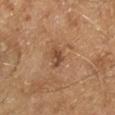Recorded during total-body skin imaging; not selected for excision or biopsy. A male subject, roughly 65 years of age. The recorded lesion diameter is about 2 mm. The total-body-photography lesion software estimated an eccentricity of roughly 0.65 and two-axis asymmetry of about 0.25. The analysis additionally found a mean CIELAB color near L≈46 a*≈20 b*≈32, a lesion–skin lightness drop of about 10, and a lesion-to-skin contrast of about 7.5 (normalized; higher = more distinct). It also reported a color-variation rating of about 3/10 and a peripheral color-asymmetry measure near 1. The software also gave a nevus-likeness score of about 5/100 and a detector confidence of about 100 out of 100 that the crop contains a lesion. A lesion tile, about 15 mm wide, cut from a 3D total-body photograph. From the leg.